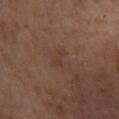The lesion was tiled from a total-body skin photograph and was not biopsied.
Longest diameter approximately 2.5 mm.
The subject is a male roughly 30 years of age.
A 15 mm close-up tile from a total-body photography series done for melanoma screening.
On the left leg.
This is a cross-polarized tile.